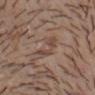Q: Was this lesion biopsied?
A: no biopsy performed (imaged during a skin exam)
Q: What did automated image analysis measure?
A: an area of roughly 5.5 mm² and a shape eccentricity near 0.8; a lesion color around L≈46 a*≈17 b*≈25 in CIELAB, about 7 CIELAB-L* units darker than the surrounding skin, and a normalized lesion–skin contrast near 5.5; a within-lesion color-variation index near 2/10 and a peripheral color-asymmetry measure near 0.5; a nevus-likeness score of about 0/100
Q: What is the imaging modality?
A: ~15 mm tile from a whole-body skin photo
Q: Patient demographics?
A: male, approximately 20 years of age
Q: What is the lesion's diameter?
A: ~3.5 mm (longest diameter)
Q: Lesion location?
A: the chest
Q: Illumination type?
A: white-light